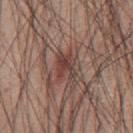A male subject aged 58–62. A 15 mm crop from a total-body photograph taken for skin-cancer surveillance. Located on the front of the torso. The lesion-visualizer software estimated a footprint of about 5.5 mm² and an eccentricity of roughly 0.65. And it measured border irregularity of about 5 on a 0–10 scale, a color-variation rating of about 3.5/10, and peripheral color asymmetry of about 1. The software also gave a nevus-likeness score of about 0/100 and lesion-presence confidence of about 95/100.